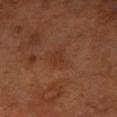Part of a total-body skin-imaging series; this lesion was reviewed on a skin check and was not flagged for biopsy.
Longest diameter approximately 2.5 mm.
Located on the left forearm.
A lesion tile, about 15 mm wide, cut from a 3D total-body photograph.
A female subject aged approximately 70.
The tile uses cross-polarized illumination.